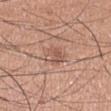Impression:
Recorded during total-body skin imaging; not selected for excision or biopsy.
Image and clinical context:
Automated image analysis of the tile measured border irregularity of about 2 on a 0–10 scale, internal color variation of about 4 on a 0–10 scale, and peripheral color asymmetry of about 1.5. And it measured a nevus-likeness score of about 0/100. A male subject aged 33–37. From the right upper arm. A 15 mm crop from a total-body photograph taken for skin-cancer surveillance. Approximately 2.5 mm at its widest.